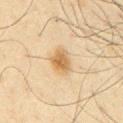Clinical impression: This lesion was catalogued during total-body skin photography and was not selected for biopsy. Context: A male subject aged 63 to 67. The lesion is located on the chest. About 3 mm across. A 15 mm crop from a total-body photograph taken for skin-cancer surveillance. Imaged with cross-polarized lighting.